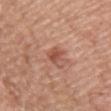| field | value |
|---|---|
| notes | imaged on a skin check; not biopsied |
| tile lighting | white-light |
| diameter | about 2.5 mm |
| automated metrics | a color-variation rating of about 1.5/10 and peripheral color asymmetry of about 0.5; a classifier nevus-likeness of about 30/100 and lesion-presence confidence of about 100/100 |
| patient | male, in their mid-70s |
| acquisition | total-body-photography crop, ~15 mm field of view |
| site | the left upper arm |